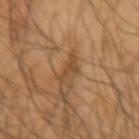<lesion>
<biopsy_status>not biopsied; imaged during a skin examination</biopsy_status>
<site>right forearm</site>
<patient>
  <sex>male</sex>
  <age_approx>65</age_approx>
</patient>
<image>
  <source>total-body photography crop</source>
  <field_of_view_mm>15</field_of_view_mm>
</image>
</lesion>